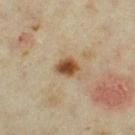Part of a total-body skin-imaging series; this lesion was reviewed on a skin check and was not flagged for biopsy. Located on the left thigh. The lesion's longest dimension is about 3 mm. Automated tile analysis of the lesion measured a footprint of about 5 mm² and a symmetry-axis asymmetry near 0.2. And it measured a border-irregularity index near 1.5/10, a color-variation rating of about 4.5/10, and a peripheral color-asymmetry measure near 1. And it measured an automated nevus-likeness rating near 100 out of 100 and a lesion-detection confidence of about 100/100. A roughly 15 mm field-of-view crop from a total-body skin photograph. A female patient, in their mid-30s. Captured under cross-polarized illumination.